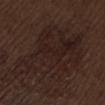Captured during whole-body skin photography for melanoma surveillance; the lesion was not biopsied. About 11 mm across. A roughly 15 mm field-of-view crop from a total-body skin photograph. Captured under white-light illumination. The lesion is located on the lower back. A male subject, aged 68–72.